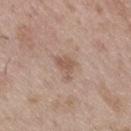  biopsy_status: not biopsied; imaged during a skin examination
  image:
    source: total-body photography crop
    field_of_view_mm: 15
  lesion_size:
    long_diameter_mm_approx: 3.0
  patient:
    sex: male
    age_approx: 50
  lighting: white-light
  site: leg